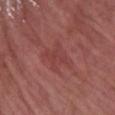This lesion was catalogued during total-body skin photography and was not selected for biopsy. A region of skin cropped from a whole-body photographic capture, roughly 15 mm wide. A female subject, approximately 60 years of age. From the right forearm. The recorded lesion diameter is about 4.5 mm.